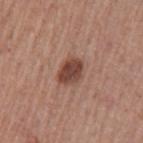<record>
  <biopsy_status>not biopsied; imaged during a skin examination</biopsy_status>
  <patient>
    <sex>male</sex>
    <age_approx>55</age_approx>
  </patient>
  <site>left upper arm</site>
  <automated_metrics>
    <vs_skin_darker_L>13.0</vs_skin_darker_L>
    <vs_skin_contrast_norm>9.5</vs_skin_contrast_norm>
    <nevus_likeness_0_100>85</nevus_likeness_0_100>
    <lesion_detection_confidence_0_100>100</lesion_detection_confidence_0_100>
  </automated_metrics>
  <image>
    <source>total-body photography crop</source>
    <field_of_view_mm>15</field_of_view_mm>
  </image>
</record>